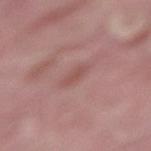Part of a total-body skin-imaging series; this lesion was reviewed on a skin check and was not flagged for biopsy. A male subject, in their 40s. A 15 mm close-up extracted from a 3D total-body photography capture. Longest diameter approximately 2.5 mm. The total-body-photography lesion software estimated an area of roughly 2.5 mm², an outline eccentricity of about 0.9 (0 = round, 1 = elongated), and a symmetry-axis asymmetry near 0.2. The software also gave a border-irregularity rating of about 3/10, a color-variation rating of about 0/10, and peripheral color asymmetry of about 0. From the lower back.